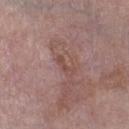Q: Is there a histopathology result?
A: catalogued during a skin exam; not biopsied
Q: Where on the body is the lesion?
A: the right lower leg
Q: What are the patient's age and sex?
A: male, roughly 85 years of age or older
Q: What is the imaging modality?
A: ~15 mm tile from a whole-body skin photo
Q: Lesion size?
A: ~3 mm (longest diameter)
Q: What lighting was used for the tile?
A: white-light